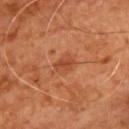| feature | finding |
|---|---|
| site | the front of the torso |
| subject | male, aged around 55 |
| tile lighting | cross-polarized |
| diameter | ≈2.5 mm |
| image | ~15 mm crop, total-body skin-cancer survey |